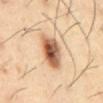Clinical impression:
The lesion was tiled from a total-body skin photograph and was not biopsied.
Clinical summary:
About 5 mm across. The patient is a male in their mid- to late 50s. A roughly 15 mm field-of-view crop from a total-body skin photograph. The lesion is on the abdomen.